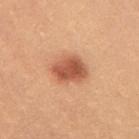Q: What did automated image analysis measure?
A: a mean CIELAB color near L≈56 a*≈28 b*≈35, roughly 14 lightness units darker than nearby skin, and a lesion-to-skin contrast of about 9 (normalized; higher = more distinct); a border-irregularity rating of about 2/10, a within-lesion color-variation index near 4/10, and a peripheral color-asymmetry measure near 1.5
Q: What is the imaging modality?
A: 15 mm crop, total-body photography
Q: Who is the patient?
A: female, aged 23 to 27
Q: Lesion size?
A: about 4 mm
Q: Lesion location?
A: the mid back